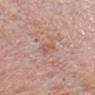Clinical impression:
Captured during whole-body skin photography for melanoma surveillance; the lesion was not biopsied.
Acquisition and patient details:
Imaged with white-light lighting. About 2.5 mm across. The patient is a male in their 80s. A roughly 15 mm field-of-view crop from a total-body skin photograph. On the head or neck.